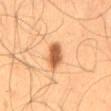The lesion was tiled from a total-body skin photograph and was not biopsied.
The recorded lesion diameter is about 5 mm.
The lesion-visualizer software estimated a footprint of about 6.5 mm² and a symmetry-axis asymmetry near 0.25. And it measured a mean CIELAB color near L≈59 a*≈25 b*≈40 and about 17 CIELAB-L* units darker than the surrounding skin. It also reported a border-irregularity rating of about 3.5/10, a color-variation rating of about 3.5/10, and a peripheral color-asymmetry measure near 1. The analysis additionally found a lesion-detection confidence of about 100/100.
From the mid back.
Cropped from a whole-body photographic skin survey; the tile spans about 15 mm.
A male patient, in their mid- to late 50s.